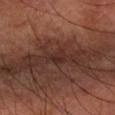follow-up: catalogued during a skin exam; not biopsied | image: ~15 mm tile from a whole-body skin photo | lesion size: ~2.5 mm (longest diameter) | site: the right forearm | lighting: cross-polarized illumination | patient: male, approximately 60 years of age.